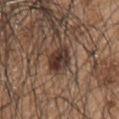Findings:
- notes — imaged on a skin check; not biopsied
- acquisition — ~15 mm tile from a whole-body skin photo
- site — the chest
- lesion diameter — about 4 mm
- subject — male, approximately 45 years of age
- illumination — white-light illumination
- image-analysis metrics — a lesion color around L≈34 a*≈17 b*≈22 in CIELAB, about 13 CIELAB-L* units darker than the surrounding skin, and a normalized border contrast of about 12; a border-irregularity rating of about 2/10, internal color variation of about 7 on a 0–10 scale, and radial color variation of about 2.5